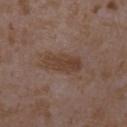Assessment:
This lesion was catalogued during total-body skin photography and was not selected for biopsy.
Background:
A female subject aged 33 to 37. From the arm. Cropped from a whole-body photographic skin survey; the tile spans about 15 mm. Longest diameter approximately 4.5 mm.